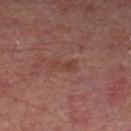{
  "biopsy_status": "not biopsied; imaged during a skin examination",
  "lesion_size": {
    "long_diameter_mm_approx": 3.0
  },
  "patient": {
    "sex": "male",
    "age_approx": 70
  },
  "site": "leg",
  "image": {
    "source": "total-body photography crop",
    "field_of_view_mm": 15
  },
  "lighting": "cross-polarized"
}